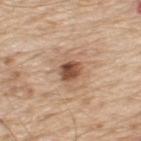Findings:
- subject — male, about 70 years old
- tile lighting — white-light
- lesion size — ~2.5 mm (longest diameter)
- body site — the upper back
- image source — ~15 mm crop, total-body skin-cancer survey